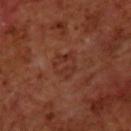Captured during whole-body skin photography for melanoma surveillance; the lesion was not biopsied. A region of skin cropped from a whole-body photographic capture, roughly 15 mm wide. This is a cross-polarized tile. The subject is a male aged around 70. The lesion is located on the upper back.